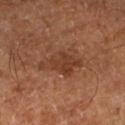Assessment:
Part of a total-body skin-imaging series; this lesion was reviewed on a skin check and was not flagged for biopsy.
Acquisition and patient details:
A patient about 65 years old. The lesion-visualizer software estimated an average lesion color of about L≈38 a*≈22 b*≈31 (CIELAB), a lesion–skin lightness drop of about 8, and a normalized border contrast of about 7. And it measured a classifier nevus-likeness of about 15/100 and lesion-presence confidence of about 100/100. The lesion is located on the left lower leg. Longest diameter approximately 5 mm. Imaged with cross-polarized lighting. Cropped from a whole-body photographic skin survey; the tile spans about 15 mm.